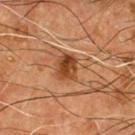Assessment:
This lesion was catalogued during total-body skin photography and was not selected for biopsy.
Image and clinical context:
A close-up tile cropped from a whole-body skin photograph, about 15 mm across. Measured at roughly 3 mm in maximum diameter. The patient is a male approximately 55 years of age. The lesion is located on the chest.